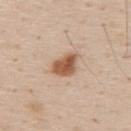| key | value |
|---|---|
| follow-up | imaged on a skin check; not biopsied |
| diameter | ≈3.5 mm |
| automated metrics | a lesion area of about 7 mm², an eccentricity of roughly 0.65, and a shape-asymmetry score of about 0.3 (0 = symmetric); an average lesion color of about L≈56 a*≈20 b*≈33 (CIELAB), a lesion–skin lightness drop of about 15, and a normalized lesion–skin contrast near 10; a border-irregularity index near 2.5/10 |
| patient | male, aged 58 to 62 |
| anatomic site | the upper back |
| image source | ~15 mm tile from a whole-body skin photo |